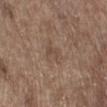Impression:
Imaged during a routine full-body skin examination; the lesion was not biopsied and no histopathology is available.
Clinical summary:
The recorded lesion diameter is about 2.5 mm. Located on the right lower leg. The tile uses white-light illumination. A 15 mm crop from a total-body photograph taken for skin-cancer surveillance. A male subject aged approximately 70. Automated image analysis of the tile measured a lesion area of about 5 mm², an eccentricity of roughly 0.65, and two-axis asymmetry of about 0.3. It also reported an average lesion color of about L≈47 a*≈15 b*≈25 (CIELAB) and about 6 CIELAB-L* units darker than the surrounding skin. The software also gave a within-lesion color-variation index near 2/10 and radial color variation of about 0.5. The analysis additionally found a classifier nevus-likeness of about 0/100 and a detector confidence of about 100 out of 100 that the crop contains a lesion.